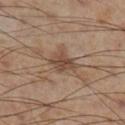Recorded during total-body skin imaging; not selected for excision or biopsy. Imaged with cross-polarized lighting. A 15 mm close-up tile from a total-body photography series done for melanoma screening. Located on the right lower leg. Automated tile analysis of the lesion measured an area of roughly 6 mm² and two-axis asymmetry of about 0.3. The software also gave a border-irregularity rating of about 3/10, internal color variation of about 3.5 on a 0–10 scale, and radial color variation of about 1. The analysis additionally found a classifier nevus-likeness of about 45/100 and lesion-presence confidence of about 100/100. A male patient, aged around 55. The lesion's longest dimension is about 3 mm.